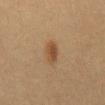The lesion was photographed on a routine skin check and not biopsied; there is no pathology result.
The lesion is located on the abdomen.
Approximately 3.5 mm at its widest.
This image is a 15 mm lesion crop taken from a total-body photograph.
A female subject aged 18 to 22.
Imaged with cross-polarized lighting.
Automated image analysis of the tile measured a lesion-to-skin contrast of about 7.5 (normalized; higher = more distinct). The analysis additionally found a border-irregularity index near 2.5/10 and a peripheral color-asymmetry measure near 1.